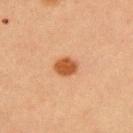No biopsy was performed on this lesion — it was imaged during a full skin examination and was not determined to be concerning.
A 15 mm close-up extracted from a 3D total-body photography capture.
Longest diameter approximately 3 mm.
This is a cross-polarized tile.
Located on the upper back.
An algorithmic analysis of the crop reported a lesion color around L≈45 a*≈23 b*≈35 in CIELAB, about 12 CIELAB-L* units darker than the surrounding skin, and a lesion-to-skin contrast of about 10 (normalized; higher = more distinct). And it measured a nevus-likeness score of about 100/100.
A male subject, approximately 60 years of age.